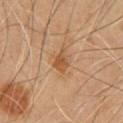Case summary:
– workup: imaged on a skin check; not biopsied
– patient: male, aged around 55
– location: the chest
– size: ~3 mm (longest diameter)
– image source: total-body-photography crop, ~15 mm field of view